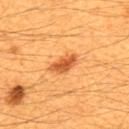Q: Is there a histopathology result?
A: imaged on a skin check; not biopsied
Q: What are the patient's age and sex?
A: male, roughly 60 years of age
Q: How was this image acquired?
A: total-body-photography crop, ~15 mm field of view
Q: Where on the body is the lesion?
A: the upper back
Q: What lighting was used for the tile?
A: cross-polarized
Q: How large is the lesion?
A: ~4 mm (longest diameter)
Q: What did automated image analysis measure?
A: a footprint of about 6 mm², a shape eccentricity near 0.85, and a symmetry-axis asymmetry near 0.25